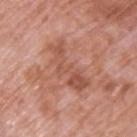Background:
On the back. A 15 mm close-up tile from a total-body photography series done for melanoma screening. An algorithmic analysis of the crop reported a footprint of about 12 mm², a shape eccentricity near 0.9, and a symmetry-axis asymmetry near 0.5. A male subject, roughly 70 years of age.